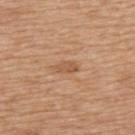Part of a total-body skin-imaging series; this lesion was reviewed on a skin check and was not flagged for biopsy. From the upper back. A lesion tile, about 15 mm wide, cut from a 3D total-body photograph. Captured under white-light illumination. About 2.5 mm across. The patient is a male aged 63 to 67.